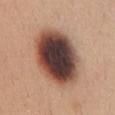{"patient": {"sex": "female", "age_approx": 30}, "lighting": "white-light", "image": {"source": "total-body photography crop", "field_of_view_mm": 15}, "automated_metrics": {"area_mm2_approx": 36.0, "eccentricity": 0.7, "cielab_L": 42, "cielab_a": 19, "cielab_b": 23, "vs_skin_darker_L": 24.0, "vs_skin_contrast_norm": 16.5, "border_irregularity_0_10": 1.0, "color_variation_0_10": 9.0, "peripheral_color_asymmetry": 2.5, "nevus_likeness_0_100": 0, "lesion_detection_confidence_0_100": 100}, "site": "chest", "lesion_size": {"long_diameter_mm_approx": 8.0}}